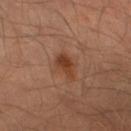Imaged during a routine full-body skin examination; the lesion was not biopsied and no histopathology is available.
The lesion is located on the left thigh.
Captured under cross-polarized illumination.
A 15 mm crop from a total-body photograph taken for skin-cancer surveillance.
A male patient aged 38–42.